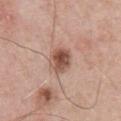No biopsy was performed on this lesion — it was imaged during a full skin examination and was not determined to be concerning. Cropped from a whole-body photographic skin survey; the tile spans about 15 mm. A male patient, approximately 60 years of age. From the chest. Approximately 3 mm at its widest. The lesion-visualizer software estimated an area of roughly 7 mm², a shape eccentricity near 0.55, and a symmetry-axis asymmetry near 0.1. And it measured a lesion–skin lightness drop of about 14 and a lesion-to-skin contrast of about 9.5 (normalized; higher = more distinct). The analysis additionally found border irregularity of about 1 on a 0–10 scale and a color-variation rating of about 6.5/10. It also reported an automated nevus-likeness rating near 90 out of 100. The tile uses white-light illumination.